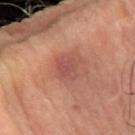The lesion was tiled from a total-body skin photograph and was not biopsied.